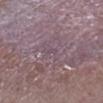<record>
<lighting>white-light</lighting>
<automated_metrics>
  <vs_skin_darker_L>4.0</vs_skin_darker_L>
</automated_metrics>
<patient>
  <sex>male</sex>
  <age_approx>75</age_approx>
</patient>
<site>right lower leg</site>
<image>
  <source>total-body photography crop</source>
  <field_of_view_mm>15</field_of_view_mm>
</image>
</record>